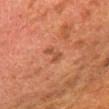Recorded during total-body skin imaging; not selected for excision or biopsy. A 15 mm close-up extracted from a 3D total-body photography capture. The recorded lesion diameter is about 3 mm. A male subject, in their 80s. From the right lower leg. This is a cross-polarized tile.